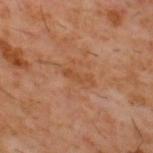From the upper back. Approximately 3 mm at its widest. A male patient aged approximately 60. The total-body-photography lesion software estimated an outline eccentricity of about 0.95 (0 = round, 1 = elongated) and a shape-asymmetry score of about 0.45 (0 = symmetric). The analysis additionally found a lesion–skin lightness drop of about 6 and a normalized border contrast of about 6. The analysis additionally found a border-irregularity rating of about 5.5/10 and peripheral color asymmetry of about 0. And it measured a classifier nevus-likeness of about 0/100 and a lesion-detection confidence of about 100/100. Cropped from a whole-body photographic skin survey; the tile spans about 15 mm.